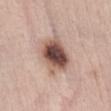workup: total-body-photography surveillance lesion; no biopsy | body site: the abdomen | image-analysis metrics: a border-irregularity rating of about 2.5/10; an automated nevus-likeness rating near 95 out of 100 | diameter: ~5 mm (longest diameter) | illumination: white-light illumination | subject: female, aged 33 to 37 | image source: total-body-photography crop, ~15 mm field of view.